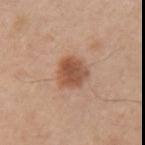Acquisition and patient details:
On the left upper arm. The subject is a male about 70 years old. Cropped from a total-body skin-imaging series; the visible field is about 15 mm.